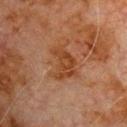  biopsy_status: not biopsied; imaged during a skin examination
  patient:
    sex: male
    age_approx: 80
  lesion_size:
    long_diameter_mm_approx: 4.5
  image:
    source: total-body photography crop
    field_of_view_mm: 15
  lighting: cross-polarized
  site: chest